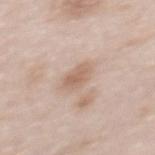No biopsy was performed on this lesion — it was imaged during a full skin examination and was not determined to be concerning. The lesion's longest dimension is about 3 mm. The total-body-photography lesion software estimated a lesion area of about 5 mm², an eccentricity of roughly 0.7, and a shape-asymmetry score of about 0.2 (0 = symmetric). It also reported a mean CIELAB color near L≈62 a*≈17 b*≈28, about 9 CIELAB-L* units darker than the surrounding skin, and a normalized lesion–skin contrast near 6. The analysis additionally found a border-irregularity rating of about 2/10, internal color variation of about 2.5 on a 0–10 scale, and peripheral color asymmetry of about 1. This image is a 15 mm lesion crop taken from a total-body photograph. A female patient in their mid- to late 60s. From the back.